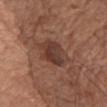Q: Was a biopsy performed?
A: imaged on a skin check; not biopsied
Q: What lighting was used for the tile?
A: white-light
Q: How was this image acquired?
A: 15 mm crop, total-body photography
Q: What are the patient's age and sex?
A: male, approximately 75 years of age
Q: What did automated image analysis measure?
A: an area of roughly 12 mm², an eccentricity of roughly 0.9, and a shape-asymmetry score of about 0.25 (0 = symmetric); a lesion color around L≈38 a*≈20 b*≈25 in CIELAB and a normalized border contrast of about 8; border irregularity of about 3 on a 0–10 scale and a within-lesion color-variation index near 6/10; lesion-presence confidence of about 100/100
Q: Where on the body is the lesion?
A: the chest